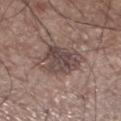Findings:
– follow-up: total-body-photography surveillance lesion; no biopsy
– subject: male, in their 60s
– location: the left lower leg
– automated lesion analysis: a border-irregularity index near 2/10, internal color variation of about 5 on a 0–10 scale, and a peripheral color-asymmetry measure near 2; an automated nevus-likeness rating near 5 out of 100 and lesion-presence confidence of about 100/100
– acquisition: 15 mm crop, total-body photography
– illumination: white-light illumination